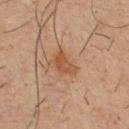notes: no biopsy performed (imaged during a skin exam)
site: the chest
patient: male, about 35 years old
illumination: cross-polarized illumination
lesion size: about 3.5 mm
image-analysis metrics: an area of roughly 6 mm², an eccentricity of roughly 0.8, and a shape-asymmetry score of about 0.35 (0 = symmetric); a border-irregularity index near 3/10 and internal color variation of about 3 on a 0–10 scale; an automated nevus-likeness rating near 75 out of 100 and a lesion-detection confidence of about 100/100
imaging modality: total-body-photography crop, ~15 mm field of view